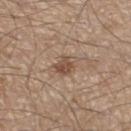This is a white-light tile. On the leg. A 15 mm close-up extracted from a 3D total-body photography capture. The total-body-photography lesion software estimated a mean CIELAB color near L≈50 a*≈17 b*≈28, roughly 10 lightness units darker than nearby skin, and a normalized lesion–skin contrast near 7.5. A male patient, aged 58–62. The lesion's longest dimension is about 3 mm.